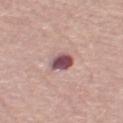Case summary:
* biopsy status: total-body-photography surveillance lesion; no biopsy
* patient: female, aged around 65
* acquisition: ~15 mm crop, total-body skin-cancer survey
* automated metrics: a footprint of about 5.5 mm² and an outline eccentricity of about 0.75 (0 = round, 1 = elongated); a lesion–skin lightness drop of about 18 and a lesion-to-skin contrast of about 13 (normalized; higher = more distinct); a nevus-likeness score of about 0/100 and a detector confidence of about 100 out of 100 that the crop contains a lesion
* illumination: white-light illumination
* body site: the left thigh